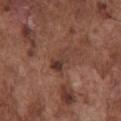| feature | finding |
|---|---|
| workup | imaged on a skin check; not biopsied |
| tile lighting | white-light illumination |
| image | total-body-photography crop, ~15 mm field of view |
| location | the chest |
| subject | male, aged approximately 75 |
| diameter | ≈3 mm |